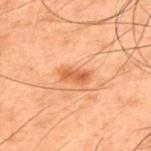| key | value |
|---|---|
| notes | imaged on a skin check; not biopsied |
| lesion size | ~3.5 mm (longest diameter) |
| lighting | cross-polarized |
| image source | 15 mm crop, total-body photography |
| anatomic site | the upper back |
| subject | male, in their 50s |
| automated lesion analysis | a lesion-detection confidence of about 100/100 |